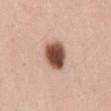This lesion was catalogued during total-body skin photography and was not selected for biopsy.